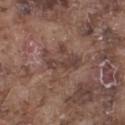biopsy status = total-body-photography surveillance lesion; no biopsy
site = the left thigh
patient = male, in their mid-70s
lesion size = about 5 mm
tile lighting = white-light illumination
imaging modality = ~15 mm crop, total-body skin-cancer survey